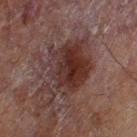The lesion was photographed on a routine skin check and not biopsied; there is no pathology result. The tile uses cross-polarized illumination. Located on the left thigh. The recorded lesion diameter is about 6.5 mm. Cropped from a whole-body photographic skin survey; the tile spans about 15 mm. The patient is a male aged 68–72. The lesion-visualizer software estimated border irregularity of about 4.5 on a 0–10 scale, a within-lesion color-variation index near 6/10, and peripheral color asymmetry of about 2.